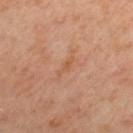Q: Was this lesion biopsied?
A: total-body-photography surveillance lesion; no biopsy
Q: Lesion location?
A: the right upper arm
Q: What kind of image is this?
A: ~15 mm crop, total-body skin-cancer survey
Q: How large is the lesion?
A: ≈3 mm
Q: What did automated image analysis measure?
A: an area of roughly 2.5 mm² and an eccentricity of roughly 0.95; a classifier nevus-likeness of about 0/100
Q: Illumination type?
A: cross-polarized illumination
Q: What are the patient's age and sex?
A: female, aged 48 to 52